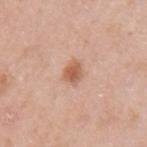Impression: Part of a total-body skin-imaging series; this lesion was reviewed on a skin check and was not flagged for biopsy.